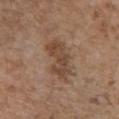{
  "biopsy_status": "not biopsied; imaged during a skin examination",
  "automated_metrics": {
    "cielab_L": 45,
    "cielab_a": 17,
    "cielab_b": 28,
    "vs_skin_darker_L": 9.0,
    "vs_skin_contrast_norm": 7.5,
    "border_irregularity_0_10": 3.5,
    "color_variation_0_10": 3.5,
    "peripheral_color_asymmetry": 1.0,
    "nevus_likeness_0_100": 0
  },
  "image": {
    "source": "total-body photography crop",
    "field_of_view_mm": 15
  },
  "lesion_size": {
    "long_diameter_mm_approx": 6.0
  },
  "patient": {
    "sex": "female",
    "age_approx": 65
  },
  "lighting": "white-light",
  "site": "chest"
}